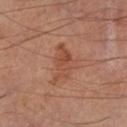| feature | finding |
|---|---|
| follow-up | catalogued during a skin exam; not biopsied |
| location | the left lower leg |
| image-analysis metrics | about 7 CIELAB-L* units darker than the surrounding skin; a border-irregularity index near 5/10 |
| acquisition | 15 mm crop, total-body photography |
| subject | male, approximately 65 years of age |
| lesion diameter | ~5 mm (longest diameter) |
| illumination | cross-polarized illumination |